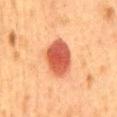Recorded during total-body skin imaging; not selected for excision or biopsy. This is a cross-polarized tile. On the mid back. The recorded lesion diameter is about 5.5 mm. The subject is a female roughly 40 years of age. A close-up tile cropped from a whole-body skin photograph, about 15 mm across.